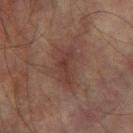Recorded during total-body skin imaging; not selected for excision or biopsy. The subject is a male in their mid-70s. A lesion tile, about 15 mm wide, cut from a 3D total-body photograph. An algorithmic analysis of the crop reported a border-irregularity rating of about 4.5/10, a within-lesion color-variation index near 3/10, and peripheral color asymmetry of about 1. The tile uses cross-polarized illumination. The lesion is located on the arm. The lesion's longest dimension is about 4.5 mm.